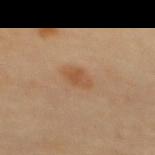Clinical summary: An algorithmic analysis of the crop reported a shape eccentricity near 0.8. Approximately 3 mm at its widest. A female patient, aged around 60. A close-up tile cropped from a whole-body skin photograph, about 15 mm across. On the mid back.